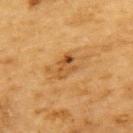Q: Is there a histopathology result?
A: imaged on a skin check; not biopsied
Q: Patient demographics?
A: male, in their mid- to late 80s or older
Q: How was this image acquired?
A: ~15 mm crop, total-body skin-cancer survey
Q: Lesion location?
A: the upper back